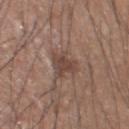Case summary:
* follow-up: imaged on a skin check; not biopsied
* TBP lesion metrics: a lesion area of about 6.5 mm² and a shape-asymmetry score of about 0.35 (0 = symmetric); an average lesion color of about L≈44 a*≈17 b*≈23 (CIELAB), a lesion–skin lightness drop of about 9, and a lesion-to-skin contrast of about 7 (normalized; higher = more distinct); a nevus-likeness score of about 0/100
* subject: male, aged 58 to 62
* image: 15 mm crop, total-body photography
* location: the head or neck
* lesion size: ≈3.5 mm